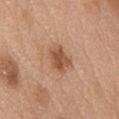{"biopsy_status": "not biopsied; imaged during a skin examination", "patient": {"sex": "female", "age_approx": 60}, "site": "abdomen", "lesion_size": {"long_diameter_mm_approx": 3.5}, "image": {"source": "total-body photography crop", "field_of_view_mm": 15}}